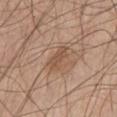Clinical impression:
Recorded during total-body skin imaging; not selected for excision or biopsy.
Context:
A lesion tile, about 15 mm wide, cut from a 3D total-body photograph. Located on the left thigh. The tile uses white-light illumination. The patient is a male aged 63 to 67.